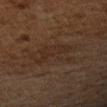Assessment:
Captured during whole-body skin photography for melanoma surveillance; the lesion was not biopsied.
Context:
Imaged with cross-polarized lighting. A male subject, in their 60s. Measured at roughly 6 mm in maximum diameter. A 15 mm close-up tile from a total-body photography series done for melanoma screening. On the left upper arm.